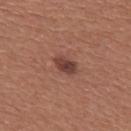Longest diameter approximately 3 mm. A 15 mm close-up tile from a total-body photography series done for melanoma screening. The tile uses white-light illumination. A female patient roughly 50 years of age. Automated tile analysis of the lesion measured a within-lesion color-variation index near 4/10 and radial color variation of about 1. The analysis additionally found an automated nevus-likeness rating near 90 out of 100 and a lesion-detection confidence of about 100/100. Located on the upper back.